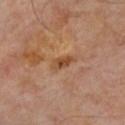{"biopsy_status": "not biopsied; imaged during a skin examination", "lighting": "cross-polarized", "site": "chest", "image": {"source": "total-body photography crop", "field_of_view_mm": 15}, "patient": {"sex": "male", "age_approx": 55}}